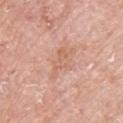{"biopsy_status": "not biopsied; imaged during a skin examination", "lesion_size": {"long_diameter_mm_approx": 4.0}, "patient": {"sex": "female", "age_approx": 65}, "site": "upper back", "image": {"source": "total-body photography crop", "field_of_view_mm": 15}}